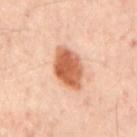| feature | finding |
|---|---|
| follow-up | total-body-photography surveillance lesion; no biopsy |
| imaging modality | ~15 mm crop, total-body skin-cancer survey |
| diameter | ~5.5 mm (longest diameter) |
| subject | roughly 55 years of age |
| location | the mid back |